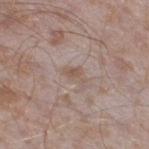No biopsy was performed on this lesion — it was imaged during a full skin examination and was not determined to be concerning. A male subject aged approximately 45. The total-body-photography lesion software estimated a lesion area of about 3 mm², an outline eccentricity of about 0.75 (0 = round, 1 = elongated), and a shape-asymmetry score of about 0.4 (0 = symmetric). The software also gave a nevus-likeness score of about 0/100 and lesion-presence confidence of about 100/100. A close-up tile cropped from a whole-body skin photograph, about 15 mm across. From the leg. The recorded lesion diameter is about 2.5 mm.